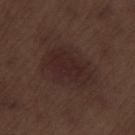  biopsy_status: not biopsied; imaged during a skin examination